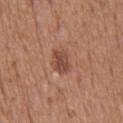Impression: Recorded during total-body skin imaging; not selected for excision or biopsy. Clinical summary: Longest diameter approximately 3.5 mm. A close-up tile cropped from a whole-body skin photograph, about 15 mm across. A male patient in their 60s. Automated image analysis of the tile measured a mean CIELAB color near L≈47 a*≈23 b*≈28, about 10 CIELAB-L* units darker than the surrounding skin, and a normalized border contrast of about 7.5. It also reported an automated nevus-likeness rating near 45 out of 100 and a lesion-detection confidence of about 100/100. This is a white-light tile. On the back.